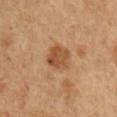workup = total-body-photography surveillance lesion; no biopsy | tile lighting = cross-polarized illumination | image source = 15 mm crop, total-body photography | subject = male, aged 48–52 | body site = the chest | size = ≈4 mm.